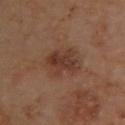Case summary:
- notes · catalogued during a skin exam; not biopsied
- image-analysis metrics · roughly 8 lightness units darker than nearby skin and a normalized border contrast of about 7; border irregularity of about 2.5 on a 0–10 scale, a within-lesion color-variation index near 5.5/10, and a peripheral color-asymmetry measure near 2; a nevus-likeness score of about 40/100 and lesion-presence confidence of about 100/100
- subject · male, in their mid-60s
- anatomic site · the back
- image source · 15 mm crop, total-body photography
- lighting · cross-polarized illumination
- lesion diameter · about 4.5 mm